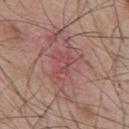biopsy_status: not biopsied; imaged during a skin examination
image:
  source: total-body photography crop
  field_of_view_mm: 15
patient:
  sex: male
  age_approx: 55
site: back
lesion_size:
  long_diameter_mm_approx: 6.0
lighting: white-light